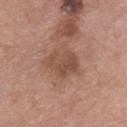Captured during whole-body skin photography for melanoma surveillance; the lesion was not biopsied. A 15 mm crop from a total-body photograph taken for skin-cancer surveillance. Captured under white-light illumination. On the front of the torso. The subject is a male in their mid-70s.